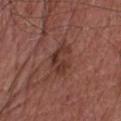<record>
  <biopsy_status>not biopsied; imaged during a skin examination</biopsy_status>
  <patient>
    <sex>male</sex>
    <age_approx>65</age_approx>
  </patient>
  <image>
    <source>total-body photography crop</source>
    <field_of_view_mm>15</field_of_view_mm>
  </image>
  <site>chest</site>
</record>